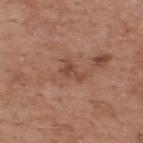No biopsy was performed on this lesion — it was imaged during a full skin examination and was not determined to be concerning. A male patient, about 55 years old. A 15 mm crop from a total-body photograph taken for skin-cancer surveillance. The lesion's longest dimension is about 3 mm. The tile uses white-light illumination. Automated tile analysis of the lesion measured a border-irregularity rating of about 5.5/10. The analysis additionally found an automated nevus-likeness rating near 0 out of 100 and a detector confidence of about 100 out of 100 that the crop contains a lesion. The lesion is located on the upper back.